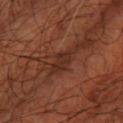Q: Is there a histopathology result?
A: no biopsy performed (imaged during a skin exam)
Q: What is the imaging modality?
A: 15 mm crop, total-body photography
Q: What did automated image analysis measure?
A: a footprint of about 3.5 mm² and an eccentricity of roughly 0.8; an average lesion color of about L≈28 a*≈22 b*≈26 (CIELAB), a lesion–skin lightness drop of about 7, and a normalized border contrast of about 6.5; an automated nevus-likeness rating near 0 out of 100 and a detector confidence of about 55 out of 100 that the crop contains a lesion
Q: Patient demographics?
A: aged approximately 65
Q: Illumination type?
A: cross-polarized illumination
Q: What is the anatomic site?
A: the right lower leg